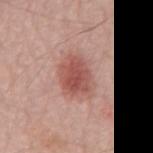Impression: Captured during whole-body skin photography for melanoma surveillance; the lesion was not biopsied. Context: A male subject approximately 70 years of age. On the mid back. A region of skin cropped from a whole-body photographic capture, roughly 15 mm wide.